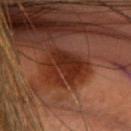Assessment:
Imaged during a routine full-body skin examination; the lesion was not biopsied and no histopathology is available.
Clinical summary:
The lesion is located on the head or neck. A 15 mm close-up extracted from a 3D total-body photography capture. A female subject aged 53–57. Captured under cross-polarized illumination.